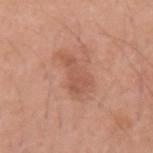lesion diameter: ~5 mm (longest diameter)
illumination: white-light
body site: the left upper arm
acquisition: ~15 mm crop, total-body skin-cancer survey
patient: male, about 30 years old